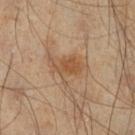biopsy status: no biopsy performed (imaged during a skin exam); location: the right lower leg; patient: male, about 45 years old; image source: ~15 mm crop, total-body skin-cancer survey.